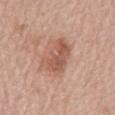Q: Is there a histopathology result?
A: total-body-photography surveillance lesion; no biopsy
Q: How large is the lesion?
A: ≈5 mm
Q: What did automated image analysis measure?
A: a footprint of about 13 mm², an eccentricity of roughly 0.8, and a symmetry-axis asymmetry near 0.2; roughly 10 lightness units darker than nearby skin and a normalized border contrast of about 7; a border-irregularity index near 2.5/10 and radial color variation of about 1.5
Q: What is the imaging modality?
A: 15 mm crop, total-body photography
Q: What are the patient's age and sex?
A: male, in their 80s
Q: Where on the body is the lesion?
A: the mid back
Q: How was the tile lit?
A: white-light illumination